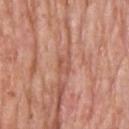Assessment:
Imaged during a routine full-body skin examination; the lesion was not biopsied and no histopathology is available.
Clinical summary:
A roughly 15 mm field-of-view crop from a total-body skin photograph. Imaged with white-light lighting. From the upper back. A male patient aged 73 to 77.